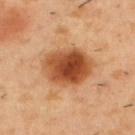Recorded during total-body skin imaging; not selected for excision or biopsy. The lesion is on the upper back. The patient is a male approximately 55 years of age. Cropped from a whole-body photographic skin survey; the tile spans about 15 mm. The lesion's longest dimension is about 5.5 mm. Imaged with cross-polarized lighting.